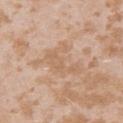<record>
<biopsy_status>not biopsied; imaged during a skin examination</biopsy_status>
<image>
  <source>total-body photography crop</source>
  <field_of_view_mm>15</field_of_view_mm>
</image>
<lighting>white-light</lighting>
<lesion_size>
  <long_diameter_mm_approx>4.5</long_diameter_mm_approx>
</lesion_size>
<patient>
  <sex>female</sex>
  <age_approx>25</age_approx>
</patient>
<automated_metrics>
  <cielab_L>63</cielab_L>
  <cielab_a>18</cielab_a>
  <cielab_b>33</cielab_b>
  <vs_skin_darker_L>6.0</vs_skin_darker_L>
  <vs_skin_contrast_norm>4.5</vs_skin_contrast_norm>
  <nevus_likeness_0_100>0</nevus_likeness_0_100>
  <lesion_detection_confidence_0_100>95</lesion_detection_confidence_0_100>
</automated_metrics>
<site>left upper arm</site>
</record>